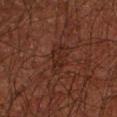No biopsy was performed on this lesion — it was imaged during a full skin examination and was not determined to be concerning. A male subject in their 50s. The total-body-photography lesion software estimated a lesion area of about 5.5 mm², a shape eccentricity near 0.85, and a symmetry-axis asymmetry near 0.35. And it measured a color-variation rating of about 1.5/10. And it measured a classifier nevus-likeness of about 0/100 and a detector confidence of about 90 out of 100 that the crop contains a lesion. Cropped from a whole-body photographic skin survey; the tile spans about 15 mm. The tile uses cross-polarized illumination. On the left forearm. Measured at roughly 3.5 mm in maximum diameter.